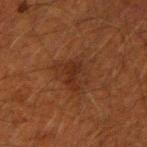Clinical impression: Captured during whole-body skin photography for melanoma surveillance; the lesion was not biopsied. Image and clinical context: Longest diameter approximately 4.5 mm. Imaged with cross-polarized lighting. A close-up tile cropped from a whole-body skin photograph, about 15 mm across. The subject is a male aged around 50. The lesion is on the right upper arm.